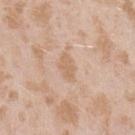  biopsy_status: not biopsied; imaged during a skin examination
  automated_metrics:
    shape_asymmetry: 0.3
    color_variation_0_10: 1.0
    peripheral_color_asymmetry: 0.5
    lesion_detection_confidence_0_100: 100
  lesion_size:
    long_diameter_mm_approx: 3.0
  lighting: white-light
  image:
    source: total-body photography crop
    field_of_view_mm: 15
  patient:
    sex: female
    age_approx: 25
  site: left upper arm